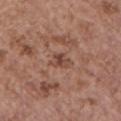Imaged during a routine full-body skin examination; the lesion was not biopsied and no histopathology is available. On the chest. The subject is a female about 65 years old. A close-up tile cropped from a whole-body skin photograph, about 15 mm across.